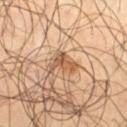Q: Was this lesion biopsied?
A: total-body-photography surveillance lesion; no biopsy
Q: What did automated image analysis measure?
A: a lesion color around L≈51 a*≈19 b*≈33 in CIELAB, about 12 CIELAB-L* units darker than the surrounding skin, and a normalized lesion–skin contrast near 8.5; a border-irregularity rating of about 6.5/10 and peripheral color asymmetry of about 1
Q: What lighting was used for the tile?
A: cross-polarized illumination
Q: How large is the lesion?
A: ≈3.5 mm
Q: Who is the patient?
A: male, approximately 55 years of age
Q: Where on the body is the lesion?
A: the leg
Q: How was this image acquired?
A: 15 mm crop, total-body photography